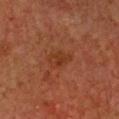{
  "biopsy_status": "not biopsied; imaged during a skin examination",
  "image": {
    "source": "total-body photography crop",
    "field_of_view_mm": 15
  },
  "site": "chest",
  "automated_metrics": {
    "border_irregularity_0_10": 2.5,
    "color_variation_0_10": 2.5,
    "peripheral_color_asymmetry": 1.0,
    "lesion_detection_confidence_0_100": 100
  },
  "patient": {
    "sex": "female",
    "age_approx": 40
  }
}